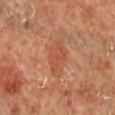Q: Was this lesion biopsied?
A: no biopsy performed (imaged during a skin exam)
Q: What are the patient's age and sex?
A: male, aged approximately 65
Q: Where on the body is the lesion?
A: the right lower leg
Q: What kind of image is this?
A: ~15 mm tile from a whole-body skin photo